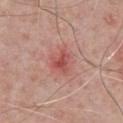Notes:
– notes — no biopsy performed (imaged during a skin exam)
– acquisition — total-body-photography crop, ~15 mm field of view
– subject — male, about 60 years old
– anatomic site — the chest